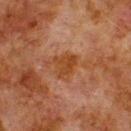The lesion was tiled from a total-body skin photograph and was not biopsied. A 15 mm close-up extracted from a 3D total-body photography capture. On the upper back. The lesion-visualizer software estimated a lesion area of about 7 mm², a shape eccentricity near 0.6, and a shape-asymmetry score of about 0.35 (0 = symmetric). It also reported border irregularity of about 3.5 on a 0–10 scale and radial color variation of about 1. Imaged with cross-polarized lighting. A male subject, aged approximately 80.